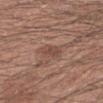notes: imaged on a skin check; not biopsied | imaging modality: total-body-photography crop, ~15 mm field of view | body site: the left forearm | lesion diameter: ≈2.5 mm | patient: male, aged approximately 30 | lighting: white-light illumination.